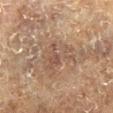Case summary:
* notes · no biopsy performed (imaged during a skin exam)
* image source · 15 mm crop, total-body photography
* lesion diameter · ~5.5 mm (longest diameter)
* body site · the right lower leg
* subject · male, in their mid- to late 70s